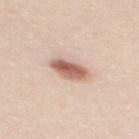The lesion was photographed on a routine skin check and not biopsied; there is no pathology result. A female patient aged 43–47. The recorded lesion diameter is about 4 mm. The lesion is on the mid back. Imaged with white-light lighting. A lesion tile, about 15 mm wide, cut from a 3D total-body photograph.